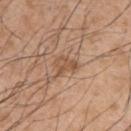{
  "biopsy_status": "not biopsied; imaged during a skin examination",
  "patient": {
    "sex": "male",
    "age_approx": 65
  },
  "lesion_size": {
    "long_diameter_mm_approx": 3.0
  },
  "image": {
    "source": "total-body photography crop",
    "field_of_view_mm": 15
  },
  "lighting": "white-light",
  "site": "arm"
}